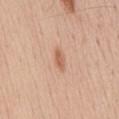Recorded during total-body skin imaging; not selected for excision or biopsy. Longest diameter approximately 2.5 mm. A male patient, in their mid- to late 50s. Captured under white-light illumination. A 15 mm close-up extracted from a 3D total-body photography capture. Located on the abdomen.